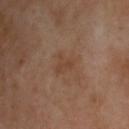workup = no biopsy performed (imaged during a skin exam)
illumination = cross-polarized
diameter = about 2.5 mm
acquisition = ~15 mm crop, total-body skin-cancer survey
location = the upper back
subject = male, aged 53–57
image-analysis metrics = a border-irregularity rating of about 4/10, internal color variation of about 1 on a 0–10 scale, and peripheral color asymmetry of about 0.5; a nevus-likeness score of about 0/100 and lesion-presence confidence of about 100/100